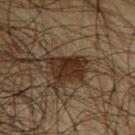Case summary:
– notes — imaged on a skin check; not biopsied
– tile lighting — cross-polarized
– subject — male, in their mid- to late 60s
– image source — total-body-photography crop, ~15 mm field of view
– automated lesion analysis — a border-irregularity index near 3.5/10
– body site — the upper back
– diameter — ~4.5 mm (longest diameter)